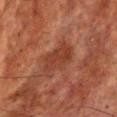The lesion was tiled from a total-body skin photograph and was not biopsied. On the front of the torso. The patient is a male approximately 65 years of age. Cropped from a whole-body photographic skin survey; the tile spans about 15 mm. Approximately 5 mm at its widest.